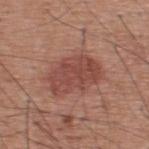No biopsy was performed on this lesion — it was imaged during a full skin examination and was not determined to be concerning.
Cropped from a whole-body photographic skin survey; the tile spans about 15 mm.
The subject is a male aged approximately 60.
Longest diameter approximately 6 mm.
From the upper back.
The tile uses white-light illumination.